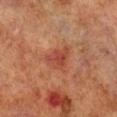Case summary:
– workup: total-body-photography surveillance lesion; no biopsy
– diameter: ~3.5 mm (longest diameter)
– anatomic site: the right lower leg
– tile lighting: cross-polarized
– subject: male, aged approximately 70
– automated metrics: a lesion area of about 6.5 mm², an outline eccentricity of about 0.75 (0 = round, 1 = elongated), and a symmetry-axis asymmetry near 0.4; a normalized border contrast of about 6.5
– imaging modality: ~15 mm tile from a whole-body skin photo